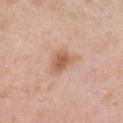Assessment:
The lesion was tiled from a total-body skin photograph and was not biopsied.
Acquisition and patient details:
This image is a 15 mm lesion crop taken from a total-body photograph. Automated image analysis of the tile measured a shape eccentricity near 0.75 and a symmetry-axis asymmetry near 0.3. It also reported a lesion color around L≈59 a*≈22 b*≈32 in CIELAB, a lesion–skin lightness drop of about 11, and a normalized lesion–skin contrast near 7.5. It also reported border irregularity of about 3 on a 0–10 scale, internal color variation of about 3 on a 0–10 scale, and radial color variation of about 1. The lesion is located on the chest. The subject is a female aged 48–52. Imaged with white-light lighting.